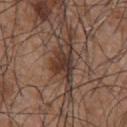<record>
  <biopsy_status>not biopsied; imaged during a skin examination</biopsy_status>
  <patient>
    <sex>male</sex>
    <age_approx>55</age_approx>
  </patient>
  <lighting>white-light</lighting>
  <image>
    <source>total-body photography crop</source>
    <field_of_view_mm>15</field_of_view_mm>
  </image>
  <site>chest</site>
  <lesion_size>
    <long_diameter_mm_approx>3.0</long_diameter_mm_approx>
  </lesion_size>
</record>